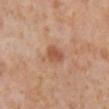Impression: The lesion was photographed on a routine skin check and not biopsied; there is no pathology result. Acquisition and patient details: The tile uses cross-polarized illumination. A region of skin cropped from a whole-body photographic capture, roughly 15 mm wide. The lesion-visualizer software estimated a footprint of about 4 mm², a shape eccentricity near 0.7, and two-axis asymmetry of about 0.2. It also reported an average lesion color of about L≈53 a*≈24 b*≈32 (CIELAB), about 10 CIELAB-L* units darker than the surrounding skin, and a normalized lesion–skin contrast near 7.5. The software also gave a border-irregularity rating of about 1.5/10, a color-variation rating of about 2.5/10, and a peripheral color-asymmetry measure near 1. Located on the left lower leg. A female subject, aged 53–57. Approximately 2.5 mm at its widest.